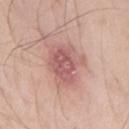Q: Is there a histopathology result?
A: no biopsy performed (imaged during a skin exam)
Q: What is the anatomic site?
A: the mid back
Q: How was this image acquired?
A: ~15 mm crop, total-body skin-cancer survey
Q: How large is the lesion?
A: about 5 mm
Q: Who is the patient?
A: male, in their 70s
Q: Automated lesion metrics?
A: border irregularity of about 3.5 on a 0–10 scale and radial color variation of about 1.5; a nevus-likeness score of about 0/100 and lesion-presence confidence of about 100/100
Q: What lighting was used for the tile?
A: white-light illumination